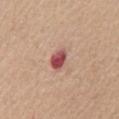Imaged during a routine full-body skin examination; the lesion was not biopsied and no histopathology is available. Imaged with white-light lighting. The lesion is located on the chest. This image is a 15 mm lesion crop taken from a total-body photograph. Approximately 3 mm at its widest. The total-body-photography lesion software estimated a lesion-to-skin contrast of about 11 (normalized; higher = more distinct). The software also gave a border-irregularity index near 1.5/10 and a color-variation rating of about 5.5/10. And it measured a nevus-likeness score of about 0/100. A male patient, aged 63–67.